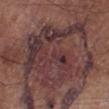Recorded during total-body skin imaging; not selected for excision or biopsy. The patient is a male aged around 55. The tile uses white-light illumination. A roughly 15 mm field-of-view crop from a total-body skin photograph. Approximately 14 mm at its widest. Located on the leg. An algorithmic analysis of the crop reported a lesion-detection confidence of about 0/100.